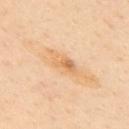Recorded during total-body skin imaging; not selected for excision or biopsy. Imaged with cross-polarized lighting. The patient is a male aged 53–57. About 5 mm across. From the upper back. Automated tile analysis of the lesion measured an outline eccentricity of about 0.9 (0 = round, 1 = elongated) and two-axis asymmetry of about 0.3. And it measured a lesion color around L≈72 a*≈19 b*≈42 in CIELAB and a normalized lesion–skin contrast near 6. A 15 mm crop from a total-body photograph taken for skin-cancer surveillance.